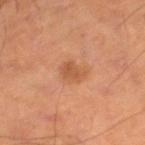Imaged with cross-polarized lighting. A male patient, aged 63–67. A close-up tile cropped from a whole-body skin photograph, about 15 mm across. Approximately 2.5 mm at its widest. Located on the left lower leg.